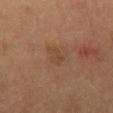This lesion was catalogued during total-body skin photography and was not selected for biopsy. A close-up tile cropped from a whole-body skin photograph, about 15 mm across. A male patient aged approximately 55. From the mid back.